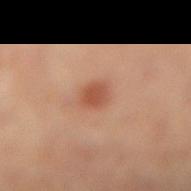* biopsy status: imaged on a skin check; not biopsied
* location: the right lower leg
* automated lesion analysis: a border-irregularity rating of about 1.5/10 and peripheral color asymmetry of about 0.5
* size: ≈2.5 mm
* illumination: cross-polarized
* imaging modality: total-body-photography crop, ~15 mm field of view
* patient: female, in their mid- to late 60s